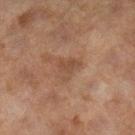follow-up: no biopsy performed (imaged during a skin exam) | patient: female, aged 58–62 | illumination: cross-polarized illumination | anatomic site: the leg | image-analysis metrics: a footprint of about 4.5 mm², a shape eccentricity near 0.55, and a shape-asymmetry score of about 0.55 (0 = symmetric); a border-irregularity rating of about 6/10, a within-lesion color-variation index near 1.5/10, and peripheral color asymmetry of about 0.5; an automated nevus-likeness rating near 0 out of 100 and a detector confidence of about 100 out of 100 that the crop contains a lesion | image source: total-body-photography crop, ~15 mm field of view | lesion diameter: ~3 mm (longest diameter).